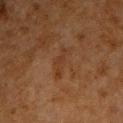Findings:
- workup · total-body-photography surveillance lesion; no biopsy
- body site · the chest
- subject · male, about 60 years old
- diameter · ≈4 mm
- lighting · cross-polarized
- acquisition · ~15 mm tile from a whole-body skin photo